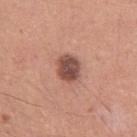Clinical impression: No biopsy was performed on this lesion — it was imaged during a full skin examination and was not determined to be concerning. Acquisition and patient details: The lesion is on the left upper arm. Captured under white-light illumination. A roughly 15 mm field-of-view crop from a total-body skin photograph. A male subject, in their 30s. Longest diameter approximately 3 mm.